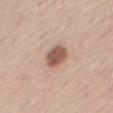Impression: Recorded during total-body skin imaging; not selected for excision or biopsy. Clinical summary: Automated tile analysis of the lesion measured a footprint of about 7 mm² and an outline eccentricity of about 0.7 (0 = round, 1 = elongated). And it measured border irregularity of about 1.5 on a 0–10 scale. The patient is a male in their mid-60s. A 15 mm close-up extracted from a 3D total-body photography capture. Longest diameter approximately 3.5 mm. The tile uses white-light illumination. The lesion is located on the right thigh.